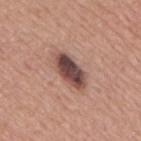No biopsy was performed on this lesion — it was imaged during a full skin examination and was not determined to be concerning.
A male patient about 75 years old.
The lesion is on the upper back.
A lesion tile, about 15 mm wide, cut from a 3D total-body photograph.
Imaged with white-light lighting.
Measured at roughly 5 mm in maximum diameter.